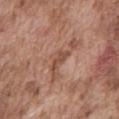Impression:
Recorded during total-body skin imaging; not selected for excision or biopsy.
Context:
The recorded lesion diameter is about 3 mm. A 15 mm close-up tile from a total-body photography series done for melanoma screening. Captured under white-light illumination. The lesion is located on the mid back. A male subject, roughly 75 years of age. An algorithmic analysis of the crop reported a lesion area of about 3.5 mm², a shape eccentricity near 0.9, and a shape-asymmetry score of about 0.5 (0 = symmetric). The software also gave an average lesion color of about L≈49 a*≈22 b*≈28 (CIELAB), about 9 CIELAB-L* units darker than the surrounding skin, and a lesion-to-skin contrast of about 7 (normalized; higher = more distinct). The analysis additionally found a nevus-likeness score of about 0/100 and lesion-presence confidence of about 75/100.